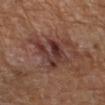The lesion was photographed on a routine skin check and not biopsied; there is no pathology result. The lesion is located on the right forearm. Measured at roughly 6 mm in maximum diameter. Cropped from a total-body skin-imaging series; the visible field is about 15 mm. Imaged with cross-polarized lighting. A male subject, aged 63–67.